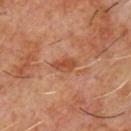Assessment:
Captured during whole-body skin photography for melanoma surveillance; the lesion was not biopsied.
Background:
The lesion is on the chest. The subject is a male aged 58–62. A 15 mm close-up tile from a total-body photography series done for melanoma screening.